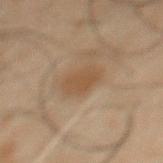Assessment: No biopsy was performed on this lesion — it was imaged during a full skin examination and was not determined to be concerning. Image and clinical context: This is a cross-polarized tile. A roughly 15 mm field-of-view crop from a total-body skin photograph. A male subject in their 50s. The lesion is on the abdomen. Automated tile analysis of the lesion measured a mean CIELAB color near L≈37 a*≈13 b*≈26, roughly 5 lightness units darker than nearby skin, and a normalized border contrast of about 6. It also reported an automated nevus-likeness rating near 15 out of 100 and a lesion-detection confidence of about 100/100.